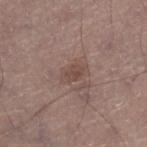Clinical impression:
Imaged during a routine full-body skin examination; the lesion was not biopsied and no histopathology is available.
Background:
About 3 mm across. A male patient, aged approximately 45. The lesion is on the right lower leg. Cropped from a total-body skin-imaging series; the visible field is about 15 mm. The total-body-photography lesion software estimated a normalized lesion–skin contrast near 6.